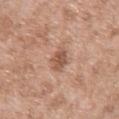biopsy status: no biopsy performed (imaged during a skin exam)
subject: male, approximately 50 years of age
anatomic site: the left upper arm
acquisition: ~15 mm crop, total-body skin-cancer survey